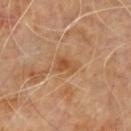This lesion was catalogued during total-body skin photography and was not selected for biopsy.
This image is a 15 mm lesion crop taken from a total-body photograph.
A male patient, about 60 years old.
On the back.
Measured at roughly 3 mm in maximum diameter.